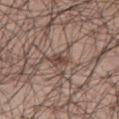biopsy_status: not biopsied; imaged during a skin examination
image:
  source: total-body photography crop
  field_of_view_mm: 15
patient:
  sex: male
  age_approx: 70
lesion_size:
  long_diameter_mm_approx: 2.5
site: left leg
lighting: white-light